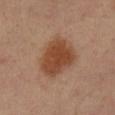{
  "automated_metrics": {
    "vs_skin_contrast_norm": 9.5,
    "border_irregularity_0_10": 2.0,
    "color_variation_0_10": 3.0,
    "peripheral_color_asymmetry": 1.0
  },
  "site": "right lower leg",
  "image": {
    "source": "total-body photography crop",
    "field_of_view_mm": 15
  },
  "lighting": "cross-polarized",
  "lesion_size": {
    "long_diameter_mm_approx": 6.0
  },
  "patient": {
    "sex": "female",
    "age_approx": 40
  }
}